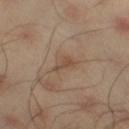Case summary:
- notes: total-body-photography surveillance lesion; no biopsy
- patient: male, approximately 45 years of age
- lighting: cross-polarized illumination
- diameter: ~2.5 mm (longest diameter)
- body site: the right thigh
- image source: total-body-photography crop, ~15 mm field of view
- automated metrics: an outline eccentricity of about 0.75 (0 = round, 1 = elongated); a mean CIELAB color near L≈46 a*≈16 b*≈27, a lesion–skin lightness drop of about 7, and a lesion-to-skin contrast of about 5.5 (normalized; higher = more distinct); a border-irregularity index near 4/10, internal color variation of about 1 on a 0–10 scale, and peripheral color asymmetry of about 0.5; a detector confidence of about 100 out of 100 that the crop contains a lesion